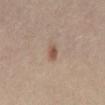lesion size: ≈2.5 mm | patient: female, about 40 years old | lighting: cross-polarized illumination | imaging modality: 15 mm crop, total-body photography | image-analysis metrics: a lesion area of about 3 mm², an eccentricity of roughly 0.75, and a shape-asymmetry score of about 0.3 (0 = symmetric); about 10 CIELAB-L* units darker than the surrounding skin; internal color variation of about 1.5 on a 0–10 scale and peripheral color asymmetry of about 0.5 | site: the abdomen.